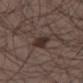Imaged during a routine full-body skin examination; the lesion was not biopsied and no histopathology is available. Measured at roughly 3 mm in maximum diameter. This image is a 15 mm lesion crop taken from a total-body photograph. Imaged with white-light lighting. From the right lower leg. A male subject aged around 40. The lesion-visualizer software estimated a lesion color around L≈30 a*≈13 b*≈18 in CIELAB. The analysis additionally found a border-irregularity index near 2/10, a within-lesion color-variation index near 2.5/10, and a peripheral color-asymmetry measure near 1. It also reported an automated nevus-likeness rating near 85 out of 100 and a detector confidence of about 100 out of 100 that the crop contains a lesion.